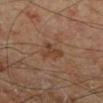The lesion was tiled from a total-body skin photograph and was not biopsied. A male patient, aged 68–72. Cropped from a whole-body photographic skin survey; the tile spans about 15 mm. About 3 mm across. Located on the left lower leg. The tile uses cross-polarized illumination.